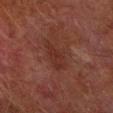Clinical impression: Recorded during total-body skin imaging; not selected for excision or biopsy. Context: The patient is a male roughly 75 years of age. Measured at roughly 4.5 mm in maximum diameter. Imaged with cross-polarized lighting. Located on the right forearm. The lesion-visualizer software estimated peripheral color asymmetry of about 0.5. The analysis additionally found a classifier nevus-likeness of about 0/100 and a detector confidence of about 95 out of 100 that the crop contains a lesion. A lesion tile, about 15 mm wide, cut from a 3D total-body photograph.